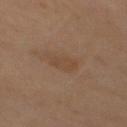  biopsy_status: not biopsied; imaged during a skin examination
  lesion_size:
    long_diameter_mm_approx: 3.5
  image:
    source: total-body photography crop
    field_of_view_mm: 15
  lighting: cross-polarized
  patient:
    sex: male
    age_approx: 70
  site: mid back
  automated_metrics:
    eccentricity: 0.8
    shape_asymmetry: 0.3
    nevus_likeness_0_100: 0
    lesion_detection_confidence_0_100: 100